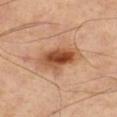Part of a total-body skin-imaging series; this lesion was reviewed on a skin check and was not flagged for biopsy. Measured at roughly 5 mm in maximum diameter. Cropped from a whole-body photographic skin survey; the tile spans about 15 mm. The lesion is located on the right thigh. Imaged with cross-polarized lighting. Automated tile analysis of the lesion measured a lesion area of about 14 mm² and two-axis asymmetry of about 0.25. And it measured a lesion color around L≈53 a*≈24 b*≈36 in CIELAB, roughly 15 lightness units darker than nearby skin, and a normalized border contrast of about 10. The analysis additionally found a detector confidence of about 100 out of 100 that the crop contains a lesion.